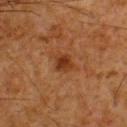| feature | finding |
|---|---|
| imaging modality | ~15 mm crop, total-body skin-cancer survey |
| patient | male, in their 60s |
| lesion diameter | ≈2.5 mm |
| anatomic site | the upper back |
| illumination | cross-polarized illumination |